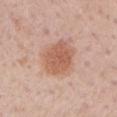Impression: Part of a total-body skin-imaging series; this lesion was reviewed on a skin check and was not flagged for biopsy. Context: Longest diameter approximately 4.5 mm. A male patient roughly 60 years of age. Cropped from a total-body skin-imaging series; the visible field is about 15 mm. The lesion is located on the left upper arm.